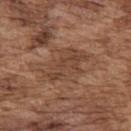Captured during whole-body skin photography for melanoma surveillance; the lesion was not biopsied. A male patient, roughly 75 years of age. Imaged with white-light lighting. On the upper back. The lesion-visualizer software estimated a footprint of about 14 mm², an outline eccentricity of about 0.85 (0 = round, 1 = elongated), and a symmetry-axis asymmetry near 0.3. It also reported roughly 7 lightness units darker than nearby skin and a normalized border contrast of about 5.5. And it measured a border-irregularity index near 4.5/10 and internal color variation of about 3 on a 0–10 scale. A region of skin cropped from a whole-body photographic capture, roughly 15 mm wide. Measured at roughly 6 mm in maximum diameter.